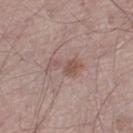Q: Was this lesion biopsied?
A: no biopsy performed (imaged during a skin exam)
Q: Where on the body is the lesion?
A: the left thigh
Q: What is the lesion's diameter?
A: ~4 mm (longest diameter)
Q: Patient demographics?
A: male, aged 53–57
Q: What is the imaging modality?
A: 15 mm crop, total-body photography
Q: What lighting was used for the tile?
A: white-light illumination